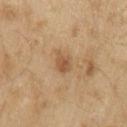workup = imaged on a skin check; not biopsied | lighting = white-light | anatomic site = the left upper arm | image source = ~15 mm crop, total-body skin-cancer survey | size = ≈2.5 mm | patient = male, aged approximately 70.